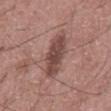Impression:
This lesion was catalogued during total-body skin photography and was not selected for biopsy.
Acquisition and patient details:
Cropped from a total-body skin-imaging series; the visible field is about 15 mm. A male subject, aged 58 to 62. Located on the abdomen.